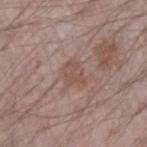biopsy status = no biopsy performed (imaged during a skin exam)
subject = male, in their 70s
automated lesion analysis = a footprint of about 3.5 mm²; a border-irregularity rating of about 4.5/10, internal color variation of about 1.5 on a 0–10 scale, and radial color variation of about 0.5
acquisition = ~15 mm tile from a whole-body skin photo
anatomic site = the left forearm